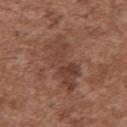Part of a total-body skin-imaging series; this lesion was reviewed on a skin check and was not flagged for biopsy. Located on the back. Cropped from a whole-body photographic skin survey; the tile spans about 15 mm. Automated image analysis of the tile measured a lesion area of about 15 mm² and two-axis asymmetry of about 0.35. The software also gave a mean CIELAB color near L≈41 a*≈21 b*≈26, a lesion–skin lightness drop of about 8, and a normalized border contrast of about 6.5. The recorded lesion diameter is about 6.5 mm. A male patient aged around 45.